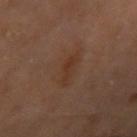A male patient, in their 70s. Automated image analysis of the tile measured border irregularity of about 5.5 on a 0–10 scale. A region of skin cropped from a whole-body photographic capture, roughly 15 mm wide. From the right upper arm. Imaged with cross-polarized lighting.